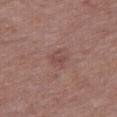Impression: Part of a total-body skin-imaging series; this lesion was reviewed on a skin check and was not flagged for biopsy. Image and clinical context: Captured under white-light illumination. Located on the right thigh. Cropped from a total-body skin-imaging series; the visible field is about 15 mm. A male patient about 75 years old. The lesion's longest dimension is about 2.5 mm.